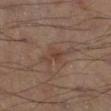The lesion was tiled from a total-body skin photograph and was not biopsied. Automated tile analysis of the lesion measured an area of roughly 6.5 mm², an eccentricity of roughly 0.9, and two-axis asymmetry of about 0.4. It also reported a lesion color around L≈38 a*≈16 b*≈23 in CIELAB and a normalized border contrast of about 5.5. The analysis additionally found a border-irregularity rating of about 6/10, a color-variation rating of about 3/10, and radial color variation of about 1. The software also gave a nevus-likeness score of about 0/100 and a lesion-detection confidence of about 100/100. A close-up tile cropped from a whole-body skin photograph, about 15 mm across. Captured under cross-polarized illumination. The lesion is located on the right lower leg. A male patient, aged around 55.